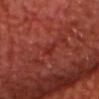Clinical impression:
This lesion was catalogued during total-body skin photography and was not selected for biopsy.
Acquisition and patient details:
Located on the chest. A male patient, aged approximately 70. Measured at roughly 2.5 mm in maximum diameter. Automated image analysis of the tile measured a lesion area of about 3 mm², a shape eccentricity near 0.85, and a shape-asymmetry score of about 0.3 (0 = symmetric). And it measured an average lesion color of about L≈30 a*≈32 b*≈28 (CIELAB) and roughly 6 lightness units darker than nearby skin. The analysis additionally found a border-irregularity rating of about 3/10, internal color variation of about 1 on a 0–10 scale, and radial color variation of about 0.5. A 15 mm crop from a total-body photograph taken for skin-cancer surveillance.